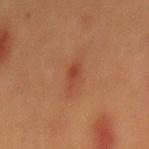notes = imaged on a skin check; not biopsied
image source = ~15 mm tile from a whole-body skin photo
site = the back
subject = male, about 40 years old